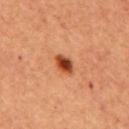The lesion was photographed on a routine skin check and not biopsied; there is no pathology result. Longest diameter approximately 3 mm. Imaged with cross-polarized lighting. A male patient roughly 65 years of age. This image is a 15 mm lesion crop taken from a total-body photograph. The lesion is located on the chest.